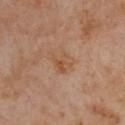  biopsy_status: not biopsied; imaged during a skin examination
  site: chest
  lighting: cross-polarized
  image:
    source: total-body photography crop
    field_of_view_mm: 15
  patient:
    sex: male
    age_approx: 55
  automated_metrics:
    eccentricity: 0.4
    lesion_detection_confidence_0_100: 100
  lesion_size:
    long_diameter_mm_approx: 3.0